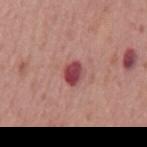Clinical impression: Imaged during a routine full-body skin examination; the lesion was not biopsied and no histopathology is available. Background: A male patient, in their 70s. Automated tile analysis of the lesion measured a lesion color around L≈45 a*≈32 b*≈20 in CIELAB and a lesion–skin lightness drop of about 15. It also reported a color-variation rating of about 4.5/10 and a peripheral color-asymmetry measure near 1.5. The software also gave a nevus-likeness score of about 0/100 and lesion-presence confidence of about 100/100. A 15 mm close-up extracted from a 3D total-body photography capture. The lesion is located on the mid back. Approximately 2.5 mm at its widest. Imaged with white-light lighting.